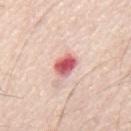Clinical impression: Part of a total-body skin-imaging series; this lesion was reviewed on a skin check and was not flagged for biopsy. Background: A 15 mm close-up extracted from a 3D total-body photography capture. The lesion is located on the mid back. The subject is a male aged 78 to 82. This is a white-light tile.